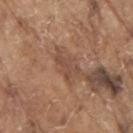Recorded during total-body skin imaging; not selected for excision or biopsy.
Imaged with white-light lighting.
A male patient aged 78–82.
Automated tile analysis of the lesion measured peripheral color asymmetry of about 0.5.
A region of skin cropped from a whole-body photographic capture, roughly 15 mm wide.
The lesion is on the right upper arm.